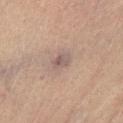Recorded during total-body skin imaging; not selected for excision or biopsy.
The recorded lesion diameter is about 2.5 mm.
A male patient, aged 58 to 62.
An algorithmic analysis of the crop reported a lesion color around L≈47 a*≈14 b*≈18 in CIELAB, a lesion–skin lightness drop of about 8, and a normalized lesion–skin contrast near 7. The analysis additionally found a border-irregularity rating of about 2.5/10 and a within-lesion color-variation index near 3/10.
A roughly 15 mm field-of-view crop from a total-body skin photograph.